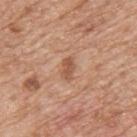Q: What kind of image is this?
A: total-body-photography crop, ~15 mm field of view
Q: How was the tile lit?
A: white-light illumination
Q: What is the lesion's diameter?
A: about 2.5 mm
Q: Patient demographics?
A: male, aged approximately 60
Q: Where on the body is the lesion?
A: the back